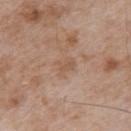{
  "biopsy_status": "not biopsied; imaged during a skin examination",
  "image": {
    "source": "total-body photography crop",
    "field_of_view_mm": 15
  },
  "patient": {
    "sex": "male",
    "age_approx": 55
  },
  "site": "upper back"
}